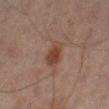{
  "biopsy_status": "not biopsied; imaged during a skin examination"
}